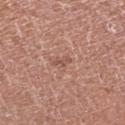<tbp_lesion>
  <biopsy_status>not biopsied; imaged during a skin examination</biopsy_status>
  <site>leg</site>
  <lighting>white-light</lighting>
  <patient>
    <sex>male</sex>
    <age_approx>65</age_approx>
  </patient>
  <lesion_size>
    <long_diameter_mm_approx>2.5</long_diameter_mm_approx>
  </lesion_size>
  <automated_metrics>
    <area_mm2_approx>1.5</area_mm2_approx>
    <eccentricity>0.95</eccentricity>
    <shape_asymmetry>0.5</shape_asymmetry>
  </automated_metrics>
  <image>
    <source>total-body photography crop</source>
    <field_of_view_mm>15</field_of_view_mm>
  </image>
</tbp_lesion>